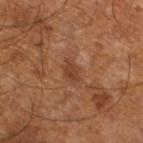<record>
<biopsy_status>not biopsied; imaged during a skin examination</biopsy_status>
<site>left lower leg</site>
<automated_metrics>
  <area_mm2_approx>3.5</area_mm2_approx>
  <eccentricity>0.8</eccentricity>
  <cielab_L>39</cielab_L>
  <cielab_a>22</cielab_a>
  <cielab_b>32</cielab_b>
  <vs_skin_darker_L>7.0</vs_skin_darker_L>
  <vs_skin_contrast_norm>6.5</vs_skin_contrast_norm>
</automated_metrics>
<patient>
  <sex>male</sex>
  <age_approx>65</age_approx>
</patient>
<lighting>cross-polarized</lighting>
<image>
  <source>total-body photography crop</source>
  <field_of_view_mm>15</field_of_view_mm>
</image>
</record>